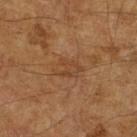Q: Was a biopsy performed?
A: imaged on a skin check; not biopsied
Q: How was this image acquired?
A: ~15 mm tile from a whole-body skin photo
Q: What are the patient's age and sex?
A: male, aged around 65
Q: Illumination type?
A: cross-polarized illumination
Q: Automated lesion metrics?
A: a lesion area of about 5.5 mm², an eccentricity of roughly 0.75, and a symmetry-axis asymmetry near 0.25; a lesion color around L≈42 a*≈20 b*≈33 in CIELAB, a lesion–skin lightness drop of about 6, and a normalized lesion–skin contrast near 4.5; a border-irregularity index near 3/10, internal color variation of about 3 on a 0–10 scale, and radial color variation of about 1; a classifier nevus-likeness of about 0/100 and lesion-presence confidence of about 95/100